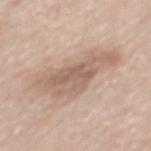Part of a total-body skin-imaging series; this lesion was reviewed on a skin check and was not flagged for biopsy. The lesion is located on the mid back. Longest diameter approximately 7.5 mm. A male subject aged 53 to 57. A 15 mm close-up extracted from a 3D total-body photography capture. The tile uses white-light illumination.